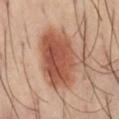The lesion was photographed on a routine skin check and not biopsied; there is no pathology result. A region of skin cropped from a whole-body photographic capture, roughly 15 mm wide. A male subject, approximately 40 years of age. Located on the abdomen.